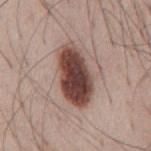Q: Is there a histopathology result?
A: catalogued during a skin exam; not biopsied
Q: What did automated image analysis measure?
A: a lesion color around L≈44 a*≈20 b*≈22 in CIELAB, about 20 CIELAB-L* units darker than the surrounding skin, and a normalized lesion–skin contrast near 14; a lesion-detection confidence of about 100/100
Q: What kind of image is this?
A: 15 mm crop, total-body photography
Q: Patient demographics?
A: male, aged 53–57
Q: How was the tile lit?
A: white-light illumination
Q: Where on the body is the lesion?
A: the mid back
Q: How large is the lesion?
A: about 7 mm